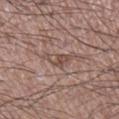workup = no biopsy performed (imaged during a skin exam).